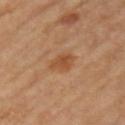Captured during whole-body skin photography for melanoma surveillance; the lesion was not biopsied. Imaged with cross-polarized lighting. The lesion is on the left upper arm. A lesion tile, about 15 mm wide, cut from a 3D total-body photograph. The subject is a female in their mid-60s.